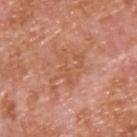Notes:
• biopsy status · no biopsy performed (imaged during a skin exam)
• image source · total-body-photography crop, ~15 mm field of view
• site · the back
• patient · male, in their mid- to late 60s
• lesion diameter · about 6 mm
• lighting · white-light illumination